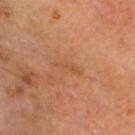Clinical impression: No biopsy was performed on this lesion — it was imaged during a full skin examination and was not determined to be concerning. Image and clinical context: The recorded lesion diameter is about 3.5 mm. A 15 mm close-up tile from a total-body photography series done for melanoma screening. The lesion is located on the head or neck. A male subject about 60 years old. Imaged with cross-polarized lighting.